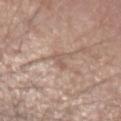biopsy status: imaged on a skin check; not biopsied | body site: the left upper arm | automated metrics: an area of roughly 2.5 mm² and an outline eccentricity of about 0.85 (0 = round, 1 = elongated); an automated nevus-likeness rating near 0 out of 100 | acquisition: 15 mm crop, total-body photography | diameter: ≈2.5 mm | patient: male, in their mid-70s.